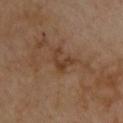Imaged during a routine full-body skin examination; the lesion was not biopsied and no histopathology is available. A close-up tile cropped from a whole-body skin photograph, about 15 mm across. The lesion is located on the upper back. A male subject, about 55 years old.